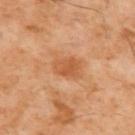| key | value |
|---|---|
| biopsy status | no biopsy performed (imaged during a skin exam) |
| anatomic site | the upper back |
| TBP lesion metrics | an area of roughly 5 mm² and a shape-asymmetry score of about 0.25 (0 = symmetric); a within-lesion color-variation index near 2/10 |
| patient | male, approximately 60 years of age |
| image | 15 mm crop, total-body photography |